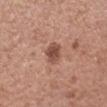Acquisition and patient details:
The subject is a female aged approximately 40. The lesion is on the left upper arm. A 15 mm crop from a total-body photograph taken for skin-cancer surveillance.